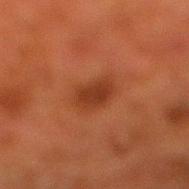workup = no biopsy performed (imaged during a skin exam)
body site = the right lower leg
subject = male, aged around 80
imaging modality = ~15 mm tile from a whole-body skin photo
tile lighting = cross-polarized